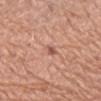Clinical impression: Captured during whole-body skin photography for melanoma surveillance; the lesion was not biopsied. Acquisition and patient details: On the left forearm. Measured at roughly 2.5 mm in maximum diameter. Captured under white-light illumination. Cropped from a whole-body photographic skin survey; the tile spans about 15 mm. A female patient in their 60s.